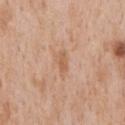- biopsy status — imaged on a skin check; not biopsied
- image source — total-body-photography crop, ~15 mm field of view
- anatomic site — the chest
- subject — male, aged approximately 65
- automated lesion analysis — border irregularity of about 4 on a 0–10 scale, a within-lesion color-variation index near 1.5/10, and a peripheral color-asymmetry measure near 0.5; a detector confidence of about 100 out of 100 that the crop contains a lesion
- size — about 3 mm